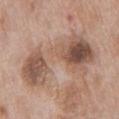Q: Was a biopsy performed?
A: no biopsy performed (imaged during a skin exam)
Q: What is the imaging modality?
A: 15 mm crop, total-body photography
Q: What did automated image analysis measure?
A: a footprint of about 36 mm², a shape eccentricity near 0.9, and two-axis asymmetry of about 0.4; a mean CIELAB color near L≈54 a*≈18 b*≈27, about 11 CIELAB-L* units darker than the surrounding skin, and a normalized lesion–skin contrast near 7.5; a classifier nevus-likeness of about 0/100 and a lesion-detection confidence of about 100/100
Q: What lighting was used for the tile?
A: white-light
Q: What is the lesion's diameter?
A: ≈10.5 mm
Q: Patient demographics?
A: male, aged around 70
Q: Where on the body is the lesion?
A: the chest